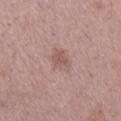The lesion was photographed on a routine skin check and not biopsied; there is no pathology result. On the leg. A lesion tile, about 15 mm wide, cut from a 3D total-body photograph. A female subject, in their 40s.